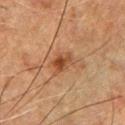  biopsy_status: not biopsied; imaged during a skin examination
  site: chest
  lighting: cross-polarized
  patient:
    sex: male
    age_approx: 50
  lesion_size:
    long_diameter_mm_approx: 3.0
  image:
    source: total-body photography crop
    field_of_view_mm: 15
  automated_metrics:
    border_irregularity_0_10: 3.5
    peripheral_color_asymmetry: 2.0
    nevus_likeness_0_100: 65
    lesion_detection_confidence_0_100: 100